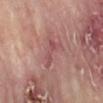subject: female, aged approximately 75; body site: the right lower leg; image: ~15 mm tile from a whole-body skin photo; TBP lesion metrics: an area of roughly 5 mm², a shape eccentricity near 0.95, and two-axis asymmetry of about 0.5; illumination: cross-polarized illumination; size: ≈4.5 mm.